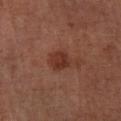follow-up: total-body-photography surveillance lesion; no biopsy | patient: male, aged around 65 | acquisition: total-body-photography crop, ~15 mm field of view | body site: the right lower leg | automated lesion analysis: a border-irregularity index near 1.5/10, internal color variation of about 2.5 on a 0–10 scale, and peripheral color asymmetry of about 1; lesion-presence confidence of about 100/100 | lighting: cross-polarized illumination | lesion size: about 2.5 mm.